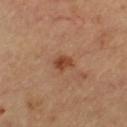{"biopsy_status": "not biopsied; imaged during a skin examination", "image": {"source": "total-body photography crop", "field_of_view_mm": 15}, "site": "left thigh", "lighting": "cross-polarized", "patient": {"sex": "female", "age_approx": 60}}